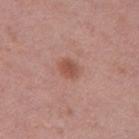Part of a total-body skin-imaging series; this lesion was reviewed on a skin check and was not flagged for biopsy. Located on the left thigh. A 15 mm close-up extracted from a 3D total-body photography capture. Imaged with white-light lighting. A female subject about 40 years old. About 2.5 mm across. The lesion-visualizer software estimated a lesion color around L≈52 a*≈24 b*≈28 in CIELAB and roughly 9 lightness units darker than nearby skin. The software also gave a border-irregularity rating of about 1.5/10, internal color variation of about 2.5 on a 0–10 scale, and a peripheral color-asymmetry measure near 1. The analysis additionally found a classifier nevus-likeness of about 90/100.